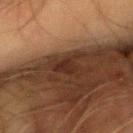patient = male, aged 63 to 67
diameter = ~3 mm (longest diameter)
anatomic site = the left forearm
imaging modality = ~15 mm crop, total-body skin-cancer survey